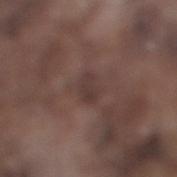Notes:
- workup · imaged on a skin check; not biopsied
- anatomic site · the leg
- image · ~15 mm crop, total-body skin-cancer survey
- subject · male, in their mid- to late 70s
- tile lighting · white-light illumination